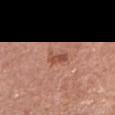Clinical impression: No biopsy was performed on this lesion — it was imaged during a full skin examination and was not determined to be concerning. Context: Cropped from a whole-body photographic skin survey; the tile spans about 15 mm. The lesion is located on the head or neck. The lesion's longest dimension is about 3 mm. Automated image analysis of the tile measured a footprint of about 3.5 mm², a shape eccentricity near 0.9, and a shape-asymmetry score of about 0.25 (0 = symmetric). The software also gave border irregularity of about 3 on a 0–10 scale, internal color variation of about 1.5 on a 0–10 scale, and radial color variation of about 0.5. It also reported a classifier nevus-likeness of about 20/100 and lesion-presence confidence of about 100/100. A female patient, in their mid- to late 70s.